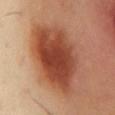This lesion was catalogued during total-body skin photography and was not selected for biopsy.